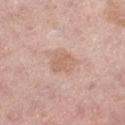Findings:
- follow-up: no biopsy performed (imaged during a skin exam)
- subject: female, aged 48–52
- acquisition: 15 mm crop, total-body photography
- tile lighting: white-light
- anatomic site: the left thigh
- lesion diameter: about 3 mm